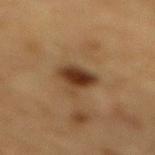Clinical impression:
Captured during whole-body skin photography for melanoma surveillance; the lesion was not biopsied.
Context:
The tile uses cross-polarized illumination. A male subject approximately 85 years of age or older. On the mid back. A roughly 15 mm field-of-view crop from a total-body skin photograph.